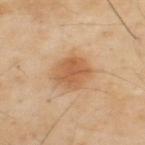Recorded during total-body skin imaging; not selected for excision or biopsy. This is a cross-polarized tile. A roughly 15 mm field-of-view crop from a total-body skin photograph. Measured at roughly 3.5 mm in maximum diameter. The patient is a male aged approximately 55. Automated tile analysis of the lesion measured a lesion color around L≈58 a*≈21 b*≈38 in CIELAB and roughly 10 lightness units darker than nearby skin. The analysis additionally found a border-irregularity rating of about 1.5/10. The lesion is on the upper back.